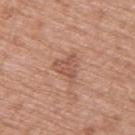biopsy status=no biopsy performed (imaged during a skin exam)
subject=male, aged around 80
image source=15 mm crop, total-body photography
tile lighting=white-light
body site=the upper back